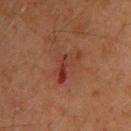workup = catalogued during a skin exam; not biopsied | patient = male, roughly 45 years of age | site = the upper back | acquisition = ~15 mm crop, total-body skin-cancer survey.